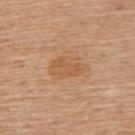Clinical impression: Captured during whole-body skin photography for melanoma surveillance; the lesion was not biopsied. Image and clinical context: Automated image analysis of the tile measured a lesion area of about 9.5 mm² and two-axis asymmetry of about 0.2. It also reported internal color variation of about 2.5 on a 0–10 scale and a peripheral color-asymmetry measure near 0.5. And it measured an automated nevus-likeness rating near 0 out of 100. The subject is a female aged 53–57. A 15 mm close-up extracted from a 3D total-body photography capture. From the upper back. Longest diameter approximately 4.5 mm.